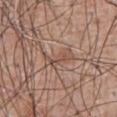Impression:
Part of a total-body skin-imaging series; this lesion was reviewed on a skin check and was not flagged for biopsy.
Acquisition and patient details:
The lesion is located on the chest. Cropped from a total-body skin-imaging series; the visible field is about 15 mm. The patient is a male aged around 60.